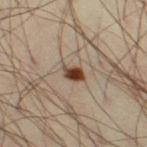<lesion>
<biopsy_status>not biopsied; imaged during a skin examination</biopsy_status>
<automated_metrics>
  <area_mm2_approx>4.0</area_mm2_approx>
  <eccentricity>0.75</eccentricity>
  <shape_asymmetry>0.2</shape_asymmetry>
  <cielab_L>41</cielab_L>
  <cielab_a>18</cielab_a>
  <cielab_b>29</cielab_b>
  <vs_skin_darker_L>17.0</vs_skin_darker_L>
  <vs_skin_contrast_norm>13.0</vs_skin_contrast_norm>
  <border_irregularity_0_10>2.0</border_irregularity_0_10>
  <color_variation_0_10>6.0</color_variation_0_10>
</automated_metrics>
<patient>
  <sex>male</sex>
  <age_approx>40</age_approx>
</patient>
<site>right thigh</site>
<image>
  <source>total-body photography crop</source>
  <field_of_view_mm>15</field_of_view_mm>
</image>
</lesion>